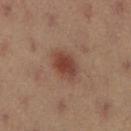No biopsy was performed on this lesion — it was imaged during a full skin examination and was not determined to be concerning.
On the right lower leg.
A female patient, aged approximately 35.
A 15 mm close-up tile from a total-body photography series done for melanoma screening.
Measured at roughly 3.5 mm in maximum diameter.